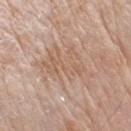Q: Is there a histopathology result?
A: catalogued during a skin exam; not biopsied
Q: What is the imaging modality?
A: 15 mm crop, total-body photography
Q: What is the anatomic site?
A: the right upper arm
Q: Who is the patient?
A: male, roughly 80 years of age